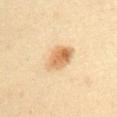- biopsy status · catalogued during a skin exam; not biopsied
- size · ≈5 mm
- image · total-body-photography crop, ~15 mm field of view
- lighting · cross-polarized
- body site · the right upper arm
- patient · male, in their mid-30s